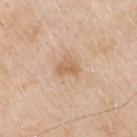{
  "biopsy_status": "not biopsied; imaged during a skin examination",
  "lesion_size": {
    "long_diameter_mm_approx": 2.5
  },
  "site": "chest",
  "automated_metrics": {
    "eccentricity": 0.8,
    "nevus_likeness_0_100": 0,
    "lesion_detection_confidence_0_100": 100
  },
  "image": {
    "source": "total-body photography crop",
    "field_of_view_mm": 15
  },
  "lighting": "white-light",
  "patient": {
    "sex": "male",
    "age_approx": 80
  }
}